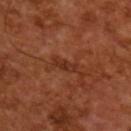{
  "biopsy_status": "not biopsied; imaged during a skin examination",
  "lesion_size": {
    "long_diameter_mm_approx": 3.0
  },
  "image": {
    "source": "total-body photography crop",
    "field_of_view_mm": 15
  },
  "automated_metrics": {
    "area_mm2_approx": 3.5,
    "eccentricity": 0.9,
    "shape_asymmetry": 0.3,
    "border_irregularity_0_10": 4.0,
    "color_variation_0_10": 0.5
  },
  "lighting": "cross-polarized",
  "patient": {
    "sex": "male",
    "age_approx": 65
  }
}